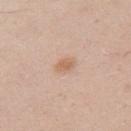Cropped from a whole-body photographic skin survey; the tile spans about 15 mm. Automated tile analysis of the lesion measured a shape eccentricity near 0.7 and a symmetry-axis asymmetry near 0.25. From the left upper arm. A male patient, roughly 30 years of age.